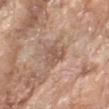| field | value |
|---|---|
| follow-up | total-body-photography surveillance lesion; no biopsy |